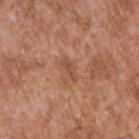<record>
<biopsy_status>not biopsied; imaged during a skin examination</biopsy_status>
<site>right upper arm</site>
<patient>
  <sex>male</sex>
  <age_approx>45</age_approx>
</patient>
<image>
  <source>total-body photography crop</source>
  <field_of_view_mm>15</field_of_view_mm>
</image>
</record>